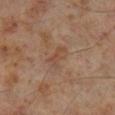The lesion was photographed on a routine skin check and not biopsied; there is no pathology result. Captured under cross-polarized illumination. Located on the right lower leg. Longest diameter approximately 3 mm. A close-up tile cropped from a whole-body skin photograph, about 15 mm across. The patient is a male aged approximately 60.